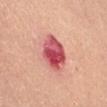<case>
  <biopsy_status>not biopsied; imaged during a skin examination</biopsy_status>
  <image>
    <source>total-body photography crop</source>
    <field_of_view_mm>15</field_of_view_mm>
  </image>
  <site>right thigh</site>
  <patient>
    <sex>female</sex>
    <age_approx>30</age_approx>
  </patient>
  <automated_metrics>
    <area_mm2_approx>13.0</area_mm2_approx>
    <eccentricity>0.75</eccentricity>
    <cielab_L>56</cielab_L>
    <cielab_a>38</cielab_a>
    <cielab_b>25</cielab_b>
    <vs_skin_darker_L>17.0</vs_skin_darker_L>
    <border_irregularity_0_10>1.5</border_irregularity_0_10>
    <peripheral_color_asymmetry>3.0</peripheral_color_asymmetry>
    <lesion_detection_confidence_0_100>100</lesion_detection_confidence_0_100>
  </automated_metrics>
  <lighting>white-light</lighting>
</case>